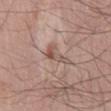<case>
<biopsy_status>not biopsied; imaged during a skin examination</biopsy_status>
<patient>
  <sex>male</sex>
  <age_approx>70</age_approx>
</patient>
<image>
  <source>total-body photography crop</source>
  <field_of_view_mm>15</field_of_view_mm>
</image>
<lighting>white-light</lighting>
<automated_metrics>
  <area_mm2_approx>4.0</area_mm2_approx>
  <eccentricity>0.95</eccentricity>
  <shape_asymmetry>0.55</shape_asymmetry>
  <border_irregularity_0_10>6.5</border_irregularity_0_10>
  <peripheral_color_asymmetry>0.5</peripheral_color_asymmetry>
  <nevus_likeness_0_100>5</nevus_likeness_0_100>
</automated_metrics>
<lesion_size>
  <long_diameter_mm_approx>4.0</long_diameter_mm_approx>
</lesion_size>
<site>mid back</site>
</case>